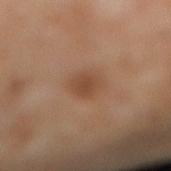Q: Is there a histopathology result?
A: catalogued during a skin exam; not biopsied
Q: What is the lesion's diameter?
A: ~3 mm (longest diameter)
Q: What are the patient's age and sex?
A: male, approximately 55 years of age
Q: Lesion location?
A: the right lower leg
Q: How was the tile lit?
A: cross-polarized illumination
Q: What kind of image is this?
A: total-body-photography crop, ~15 mm field of view
Q: What did automated image analysis measure?
A: a lesion color around L≈45 a*≈20 b*≈31 in CIELAB, a lesion–skin lightness drop of about 8, and a normalized border contrast of about 6.5; a border-irregularity rating of about 2/10 and a peripheral color-asymmetry measure near 0.5; a nevus-likeness score of about 45/100 and a lesion-detection confidence of about 100/100